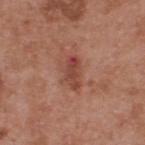No biopsy was performed on this lesion — it was imaged during a full skin examination and was not determined to be concerning. Approximately 4 mm at its widest. A 15 mm close-up tile from a total-body photography series done for melanoma screening. Imaged with white-light lighting. A male patient roughly 55 years of age. From the upper back.